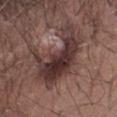Q: Is there a histopathology result?
A: total-body-photography surveillance lesion; no biopsy
Q: How was this image acquired?
A: ~15 mm tile from a whole-body skin photo
Q: Illumination type?
A: white-light illumination
Q: Automated lesion metrics?
A: an area of roughly 23 mm² and an outline eccentricity of about 0.85 (0 = round, 1 = elongated); an average lesion color of about L≈34 a*≈18 b*≈18 (CIELAB), a lesion–skin lightness drop of about 12, and a normalized border contrast of about 11; an automated nevus-likeness rating near 30 out of 100
Q: What are the patient's age and sex?
A: female, aged approximately 25
Q: What is the lesion's diameter?
A: about 7.5 mm
Q: Lesion location?
A: the abdomen